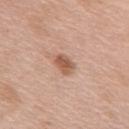The lesion was photographed on a routine skin check and not biopsied; there is no pathology result. The subject is a female about 75 years old. Cropped from a whole-body photographic skin survey; the tile spans about 15 mm. Measured at roughly 3 mm in maximum diameter. Captured under white-light illumination. Located on the mid back.